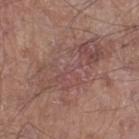This lesion was catalogued during total-body skin photography and was not selected for biopsy. This is a white-light tile. A close-up tile cropped from a whole-body skin photograph, about 15 mm across. The lesion is located on the left thigh. Measured at roughly 12 mm in maximum diameter. A male patient, approximately 60 years of age.